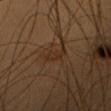Notes:
– notes — imaged on a skin check; not biopsied
– TBP lesion metrics — border irregularity of about 4 on a 0–10 scale, internal color variation of about 1 on a 0–10 scale, and a peripheral color-asymmetry measure near 0
– image source — 15 mm crop, total-body photography
– location — the head or neck
– tile lighting — cross-polarized
– subject — female, aged approximately 20
– diameter — about 2.5 mm